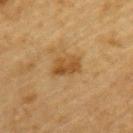Context:
A roughly 15 mm field-of-view crop from a total-body skin photograph. A male patient, roughly 85 years of age or older. The lesion is on the upper back. The recorded lesion diameter is about 3.5 mm. Imaged with cross-polarized lighting. Automated image analysis of the tile measured a lesion area of about 7.5 mm², an outline eccentricity of about 0.8 (0 = round, 1 = elongated), and a symmetry-axis asymmetry near 0.25.